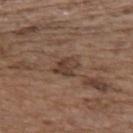Clinical summary:
A region of skin cropped from a whole-body photographic capture, roughly 15 mm wide. Located on the back. Longest diameter approximately 3 mm. Imaged with white-light lighting. The subject is a female aged 63 to 67.